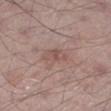Assessment: No biopsy was performed on this lesion — it was imaged during a full skin examination and was not determined to be concerning. Image and clinical context: A male subject, aged 53–57. From the left lower leg. A 15 mm close-up extracted from a 3D total-body photography capture. The lesion's longest dimension is about 3.5 mm.